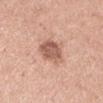Findings:
– follow-up · catalogued during a skin exam; not biopsied
– diameter · about 4 mm
– subject · male, aged around 25
– acquisition · total-body-photography crop, ~15 mm field of view
– tile lighting · white-light
– anatomic site · the right upper arm